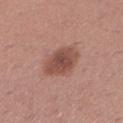workup = imaged on a skin check; not biopsied | subject = female, about 30 years old | anatomic site = the left lower leg | image = total-body-photography crop, ~15 mm field of view.